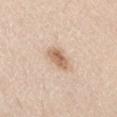Impression: Recorded during total-body skin imaging; not selected for excision or biopsy. Clinical summary: Measured at roughly 3 mm in maximum diameter. A region of skin cropped from a whole-body photographic capture, roughly 15 mm wide. Automated tile analysis of the lesion measured a shape eccentricity near 0.75 and a shape-asymmetry score of about 0.25 (0 = symmetric). The analysis additionally found a border-irregularity rating of about 2.5/10, internal color variation of about 4 on a 0–10 scale, and radial color variation of about 1.5. The software also gave an automated nevus-likeness rating near 90 out of 100 and a detector confidence of about 100 out of 100 that the crop contains a lesion. The subject is a male aged approximately 60. From the right thigh. Imaged with white-light lighting.